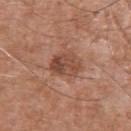<lesion>
  <biopsy_status>not biopsied; imaged during a skin examination</biopsy_status>
  <patient>
    <sex>male</sex>
    <age_approx>75</age_approx>
  </patient>
  <automated_metrics>
    <cielab_L>47</cielab_L>
    <cielab_a>22</cielab_a>
    <cielab_b>28</cielab_b>
    <border_irregularity_0_10>2.0</border_irregularity_0_10>
    <color_variation_0_10>6.0</color_variation_0_10>
    <peripheral_color_asymmetry>2.5</peripheral_color_asymmetry>
  </automated_metrics>
  <lesion_size>
    <long_diameter_mm_approx>3.5</long_diameter_mm_approx>
  </lesion_size>
  <site>arm</site>
  <image>
    <source>total-body photography crop</source>
    <field_of_view_mm>15</field_of_view_mm>
  </image>
</lesion>